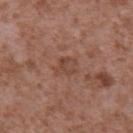Assessment: Recorded during total-body skin imaging; not selected for excision or biopsy. Context: On the upper back. Automated tile analysis of the lesion measured an average lesion color of about L≈45 a*≈21 b*≈27 (CIELAB), about 6 CIELAB-L* units darker than the surrounding skin, and a normalized border contrast of about 5. It also reported a border-irregularity rating of about 2.5/10 and radial color variation of about 1. A close-up tile cropped from a whole-body skin photograph, about 15 mm across. Captured under white-light illumination. A male patient, in their mid- to late 40s. The lesion's longest dimension is about 3 mm.